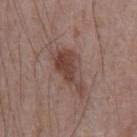The lesion is located on the chest. About 5.5 mm across. This is a white-light tile. A close-up tile cropped from a whole-body skin photograph, about 15 mm across. A male patient aged 68 to 72.